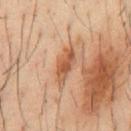Assessment: Part of a total-body skin-imaging series; this lesion was reviewed on a skin check and was not flagged for biopsy. Context: The lesion is on the chest. Cropped from a total-body skin-imaging series; the visible field is about 15 mm. A male patient about 35 years old.